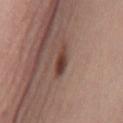| feature | finding |
|---|---|
| body site | the mid back |
| lighting | white-light illumination |
| image | 15 mm crop, total-body photography |
| TBP lesion metrics | a lesion-to-skin contrast of about 9 (normalized; higher = more distinct); a nevus-likeness score of about 100/100 and a lesion-detection confidence of about 100/100 |
| lesion diameter | ≈4 mm |
| subject | female, aged 63–67 |